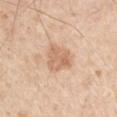Impression:
Recorded during total-body skin imaging; not selected for excision or biopsy.
Clinical summary:
The lesion's longest dimension is about 4 mm. An algorithmic analysis of the crop reported an area of roughly 8.5 mm² and a symmetry-axis asymmetry near 0.25. The analysis additionally found a mean CIELAB color near L≈66 a*≈20 b*≈33, a lesion–skin lightness drop of about 10, and a lesion-to-skin contrast of about 6 (normalized; higher = more distinct). The software also gave a border-irregularity index near 3/10, a color-variation rating of about 3.5/10, and a peripheral color-asymmetry measure near 1. The analysis additionally found a detector confidence of about 100 out of 100 that the crop contains a lesion. A 15 mm close-up extracted from a 3D total-body photography capture. A male patient approximately 55 years of age. Located on the arm. This is a white-light tile.